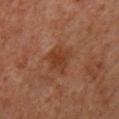No biopsy was performed on this lesion — it was imaged during a full skin examination and was not determined to be concerning. A male patient aged 58–62. A region of skin cropped from a whole-body photographic capture, roughly 15 mm wide. On the upper back. Captured under cross-polarized illumination.